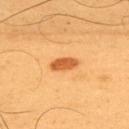<case>
  <biopsy_status>not biopsied; imaged during a skin examination</biopsy_status>
  <site>upper back</site>
  <lighting>cross-polarized</lighting>
  <image>
    <source>total-body photography crop</source>
    <field_of_view_mm>15</field_of_view_mm>
  </image>
  <lesion_size>
    <long_diameter_mm_approx>3.5</long_diameter_mm_approx>
  </lesion_size>
  <patient>
    <sex>male</sex>
    <age_approx>55</age_approx>
  </patient>
  <automated_metrics>
    <area_mm2_approx>4.5</area_mm2_approx>
    <eccentricity>0.9</eccentricity>
    <shape_asymmetry>0.25</shape_asymmetry>
    <border_irregularity_0_10>2.5</border_irregularity_0_10>
    <color_variation_0_10>2.0</color_variation_0_10>
    <peripheral_color_asymmetry>1.0</peripheral_color_asymmetry>
    <nevus_likeness_0_100>100</nevus_likeness_0_100>
    <lesion_detection_confidence_0_100>100</lesion_detection_confidence_0_100>
  </automated_metrics>
</case>